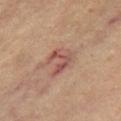The lesion was photographed on a routine skin check and not biopsied; there is no pathology result.
The tile uses cross-polarized illumination.
The subject is a female roughly 65 years of age.
On the left leg.
A lesion tile, about 15 mm wide, cut from a 3D total-body photograph.
Automated image analysis of the tile measured a footprint of about 7.5 mm², an outline eccentricity of about 0.6 (0 = round, 1 = elongated), and a shape-asymmetry score of about 0.45 (0 = symmetric). The analysis additionally found a mean CIELAB color near L≈49 a*≈22 b*≈24 and a lesion–skin lightness drop of about 9.
Measured at roughly 3.5 mm in maximum diameter.